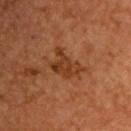Clinical impression: No biopsy was performed on this lesion — it was imaged during a full skin examination and was not determined to be concerning. Clinical summary: Approximately 4.5 mm at its widest. A roughly 15 mm field-of-view crop from a total-body skin photograph. The subject is a male about 55 years old. The lesion is located on the front of the torso.